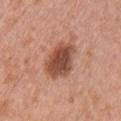<lesion>
  <biopsy_status>not biopsied; imaged during a skin examination</biopsy_status>
  <patient>
    <sex>male</sex>
    <age_approx>30</age_approx>
  </patient>
  <lesion_size>
    <long_diameter_mm_approx>5.0</long_diameter_mm_approx>
  </lesion_size>
  <automated_metrics>
    <area_mm2_approx>13.0</area_mm2_approx>
    <eccentricity>0.7</eccentricity>
    <shape_asymmetry>0.2</shape_asymmetry>
  </automated_metrics>
  <lighting>white-light</lighting>
  <site>chest</site>
  <image>
    <source>total-body photography crop</source>
    <field_of_view_mm>15</field_of_view_mm>
  </image>
</lesion>